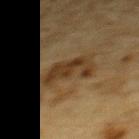Impression: Imaged during a routine full-body skin examination; the lesion was not biopsied and no histopathology is available. Clinical summary: The subject is a male aged around 85 or older. Measured at roughly 5 mm in maximum diameter. A 15 mm crop from a total-body photograph taken for skin-cancer surveillance. From the upper back. Automated tile analysis of the lesion measured a footprint of about 12 mm², an eccentricity of roughly 0.85, and two-axis asymmetry of about 0.35. The software also gave a border-irregularity index near 4.5/10 and a peripheral color-asymmetry measure near 1. The tile uses cross-polarized illumination.